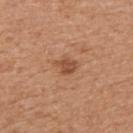{
  "biopsy_status": "not biopsied; imaged during a skin examination",
  "lighting": "white-light",
  "image": {
    "source": "total-body photography crop",
    "field_of_view_mm": 15
  },
  "patient": {
    "sex": "male",
    "age_approx": 55
  },
  "lesion_size": {
    "long_diameter_mm_approx": 2.5
  },
  "site": "right upper arm"
}